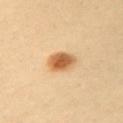The total-body-photography lesion software estimated an area of roughly 6.5 mm². And it measured a lesion-to-skin contrast of about 10 (normalized; higher = more distinct). The analysis additionally found lesion-presence confidence of about 100/100. The lesion is located on the chest. The lesion's longest dimension is about 3.5 mm. Cropped from a whole-body photographic skin survey; the tile spans about 15 mm. A female subject roughly 35 years of age. This is a cross-polarized tile.